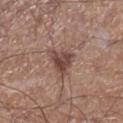Impression:
The lesion was photographed on a routine skin check and not biopsied; there is no pathology result.
Context:
A male subject roughly 60 years of age. Located on the right lower leg. The lesion-visualizer software estimated a footprint of about 7 mm², a shape eccentricity near 0.5, and a symmetry-axis asymmetry near 0.4. And it measured a mean CIELAB color near L≈45 a*≈19 b*≈23, about 12 CIELAB-L* units darker than the surrounding skin, and a lesion-to-skin contrast of about 9 (normalized; higher = more distinct). The analysis additionally found a border-irregularity index near 4/10 and radial color variation of about 1.5. And it measured a nevus-likeness score of about 70/100 and a detector confidence of about 100 out of 100 that the crop contains a lesion. A region of skin cropped from a whole-body photographic capture, roughly 15 mm wide.